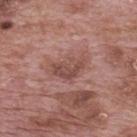workup: catalogued during a skin exam; not biopsied
tile lighting: white-light illumination
diameter: ~4.5 mm (longest diameter)
automated lesion analysis: a footprint of about 7.5 mm², a shape eccentricity near 0.85, and a shape-asymmetry score of about 0.4 (0 = symmetric); a lesion color around L≈48 a*≈22 b*≈23 in CIELAB, roughly 9 lightness units darker than nearby skin, and a normalized lesion–skin contrast near 6.5; border irregularity of about 4.5 on a 0–10 scale, a color-variation rating of about 3/10, and radial color variation of about 1; a classifier nevus-likeness of about 0/100 and a detector confidence of about 95 out of 100 that the crop contains a lesion
patient: male, aged around 70
location: the mid back
image source: ~15 mm tile from a whole-body skin photo